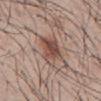Part of a total-body skin-imaging series; this lesion was reviewed on a skin check and was not flagged for biopsy. A male patient about 45 years old. A 15 mm close-up extracted from a 3D total-body photography capture. Located on the mid back.